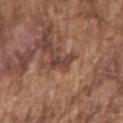No biopsy was performed on this lesion — it was imaged during a full skin examination and was not determined to be concerning.
A male subject, roughly 75 years of age.
About 3 mm across.
Cropped from a total-body skin-imaging series; the visible field is about 15 mm.
Automated tile analysis of the lesion measured an area of roughly 3 mm², an eccentricity of roughly 0.95, and a symmetry-axis asymmetry near 0.35.
The lesion is located on the right upper arm.